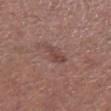Assessment: Captured during whole-body skin photography for melanoma surveillance; the lesion was not biopsied. Clinical summary: A 15 mm close-up extracted from a 3D total-body photography capture. A female subject, aged 48 to 52. On the leg.